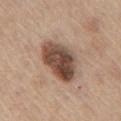workup = imaged on a skin check; not biopsied
acquisition = 15 mm crop, total-body photography
subject = male, aged 63 to 67
site = the front of the torso